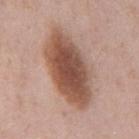Notes:
- follow-up: catalogued during a skin exam; not biopsied
- body site: the mid back
- subject: male, roughly 50 years of age
- lighting: white-light
- diameter: ≈8.5 mm
- acquisition: ~15 mm crop, total-body skin-cancer survey